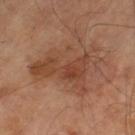anatomic site: the left thigh
illumination: cross-polarized
image source: ~15 mm tile from a whole-body skin photo
image-analysis metrics: a lesion area of about 28 mm², an eccentricity of roughly 0.75, and a shape-asymmetry score of about 0.4 (0 = symmetric); an average lesion color of about L≈43 a*≈21 b*≈30 (CIELAB), roughly 8 lightness units darker than nearby skin, and a normalized border contrast of about 6.5; border irregularity of about 6.5 on a 0–10 scale and a within-lesion color-variation index near 5.5/10; a classifier nevus-likeness of about 15/100 and lesion-presence confidence of about 100/100
patient: male, aged 68 to 72
lesion diameter: ≈8 mm